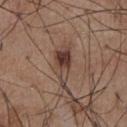<record>
<biopsy_status>not biopsied; imaged during a skin examination</biopsy_status>
<image>
  <source>total-body photography crop</source>
  <field_of_view_mm>15</field_of_view_mm>
</image>
<site>abdomen</site>
<automated_metrics>
  <border_irregularity_0_10>6.5</border_irregularity_0_10>
  <color_variation_0_10>4.0</color_variation_0_10>
  <peripheral_color_asymmetry>1.5</peripheral_color_asymmetry>
  <nevus_likeness_0_100>75</nevus_likeness_0_100>
</automated_metrics>
<patient>
  <sex>male</sex>
  <age_approx>55</age_approx>
</patient>
<lighting>white-light</lighting>
</record>